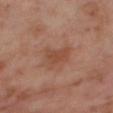Recorded during total-body skin imaging; not selected for excision or biopsy. The lesion's longest dimension is about 3.5 mm. The lesion is located on the right thigh. The total-body-photography lesion software estimated an outline eccentricity of about 0.75 (0 = round, 1 = elongated) and two-axis asymmetry of about 0.35. It also reported a lesion color around L≈48 a*≈23 b*≈31 in CIELAB, about 7 CIELAB-L* units darker than the surrounding skin, and a normalized border contrast of about 6. It also reported a nevus-likeness score of about 0/100. A close-up tile cropped from a whole-body skin photograph, about 15 mm across. A female subject, aged 53–57. This is a cross-polarized tile.